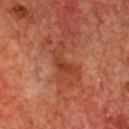biopsy status: catalogued during a skin exam; not biopsied.